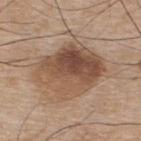Q: Was this lesion biopsied?
A: no biopsy performed (imaged during a skin exam)
Q: What kind of image is this?
A: 15 mm crop, total-body photography
Q: What are the patient's age and sex?
A: male, about 75 years old
Q: Lesion size?
A: ≈7.5 mm
Q: What lighting was used for the tile?
A: white-light illumination
Q: What is the anatomic site?
A: the upper back